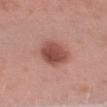<case>
  <patient>
    <sex>male</sex>
    <age_approx>55</age_approx>
  </patient>
  <image>
    <source>total-body photography crop</source>
    <field_of_view_mm>15</field_of_view_mm>
  </image>
  <site>left upper arm</site>
</case>